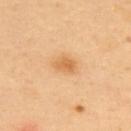No biopsy was performed on this lesion — it was imaged during a full skin examination and was not determined to be concerning. Longest diameter approximately 2.5 mm. Captured under cross-polarized illumination. This image is a 15 mm lesion crop taken from a total-body photograph. The lesion is on the back. A male patient, aged around 40.